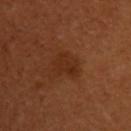| feature | finding |
|---|---|
| biopsy status | catalogued during a skin exam; not biopsied |
| patient | female, in their 50s |
| lesion diameter | ≈3.5 mm |
| TBP lesion metrics | an eccentricity of roughly 0.7; a lesion color around L≈29 a*≈23 b*≈31 in CIELAB, about 6 CIELAB-L* units darker than the surrounding skin, and a normalized border contrast of about 6; a classifier nevus-likeness of about 0/100 |
| image source | ~15 mm crop, total-body skin-cancer survey |